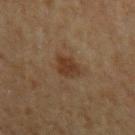Assessment:
Part of a total-body skin-imaging series; this lesion was reviewed on a skin check and was not flagged for biopsy.
Clinical summary:
A male subject about 65 years old. Automated image analysis of the tile measured a symmetry-axis asymmetry near 0.25. A 15 mm close-up extracted from a 3D total-body photography capture. Imaged with cross-polarized lighting. Located on the left upper arm. The recorded lesion diameter is about 3 mm.